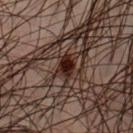follow-up=imaged on a skin check; not biopsied
TBP lesion metrics=an average lesion color of about L≈20 a*≈19 b*≈21 (CIELAB), a lesion–skin lightness drop of about 13, and a normalized lesion–skin contrast near 14.5; border irregularity of about 2.5 on a 0–10 scale and radial color variation of about 1.5; a classifier nevus-likeness of about 100/100 and a detector confidence of about 100 out of 100 that the crop contains a lesion
body site=the front of the torso
lesion diameter=about 2.5 mm
illumination=cross-polarized
patient=male, in their mid-40s
image=total-body-photography crop, ~15 mm field of view